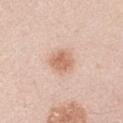Captured during whole-body skin photography for melanoma surveillance; the lesion was not biopsied. Cropped from a whole-body photographic skin survey; the tile spans about 15 mm. The lesion is located on the right upper arm. A male patient about 45 years old.